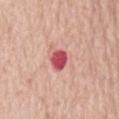Assessment:
The lesion was tiled from a total-body skin photograph and was not biopsied.
Acquisition and patient details:
Captured under white-light illumination. On the abdomen. A close-up tile cropped from a whole-body skin photograph, about 15 mm across. Longest diameter approximately 2.5 mm. A female patient, aged around 75.